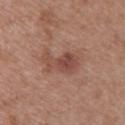Part of a total-body skin-imaging series; this lesion was reviewed on a skin check and was not flagged for biopsy. The subject is a female aged 28–32. A roughly 15 mm field-of-view crop from a total-body skin photograph. Located on the upper back.